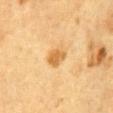biopsy status: imaged on a skin check; not biopsied
lesion size: about 2.5 mm
illumination: cross-polarized
image source: total-body-photography crop, ~15 mm field of view
subject: male, roughly 85 years of age or older
body site: the abdomen
image-analysis metrics: a lesion area of about 5 mm², an eccentricity of roughly 0.6, and two-axis asymmetry of about 0.2; roughly 10 lightness units darker than nearby skin and a normalized lesion–skin contrast near 7.5; border irregularity of about 2 on a 0–10 scale, internal color variation of about 2 on a 0–10 scale, and peripheral color asymmetry of about 1; a classifier nevus-likeness of about 50/100 and a detector confidence of about 100 out of 100 that the crop contains a lesion